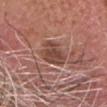Recorded during total-body skin imaging; not selected for excision or biopsy.
Located on the head or neck.
A male patient aged 73 to 77.
The lesion's longest dimension is about 4 mm.
A 15 mm close-up tile from a total-body photography series done for melanoma screening.